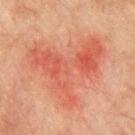Part of a total-body skin-imaging series; this lesion was reviewed on a skin check and was not flagged for biopsy. A 15 mm close-up extracted from a 3D total-body photography capture. Automated tile analysis of the lesion measured an area of roughly 32 mm², an outline eccentricity of about 0.65 (0 = round, 1 = elongated), and a symmetry-axis asymmetry near 0.7. The software also gave a lesion–skin lightness drop of about 7 and a normalized border contrast of about 6. The analysis additionally found a border-irregularity rating of about 10/10 and radial color variation of about 1.5. The analysis additionally found a classifier nevus-likeness of about 0/100 and a lesion-detection confidence of about 100/100. The tile uses cross-polarized illumination. A male subject, in their mid-70s. From the chest. Longest diameter approximately 9 mm.